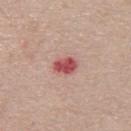Impression: The lesion was photographed on a routine skin check and not biopsied; there is no pathology result. Context: Cropped from a whole-body photographic skin survey; the tile spans about 15 mm. A female patient roughly 55 years of age. The lesion is located on the upper back. The tile uses white-light illumination.